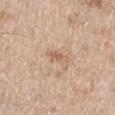* workup: catalogued during a skin exam; not biopsied
* subject: female, in their 70s
* location: the leg
* acquisition: total-body-photography crop, ~15 mm field of view